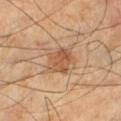Clinical impression: The lesion was photographed on a routine skin check and not biopsied; there is no pathology result. Image and clinical context: On the leg. Cropped from a whole-body photographic skin survey; the tile spans about 15 mm. Automated image analysis of the tile measured a lesion area of about 8 mm² and a shape eccentricity near 0.4. The software also gave a mean CIELAB color near L≈52 a*≈21 b*≈34, a lesion–skin lightness drop of about 9, and a lesion-to-skin contrast of about 7 (normalized; higher = more distinct). And it measured a border-irregularity rating of about 2.5/10, internal color variation of about 3.5 on a 0–10 scale, and radial color variation of about 1. The software also gave lesion-presence confidence of about 100/100. Imaged with cross-polarized lighting. A male subject, approximately 55 years of age. The recorded lesion diameter is about 3.5 mm.